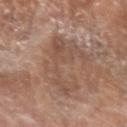| feature | finding |
|---|---|
| notes | catalogued during a skin exam; not biopsied |
| location | the left forearm |
| lesion size | ~7.5 mm (longest diameter) |
| subject | female, aged around 75 |
| illumination | white-light |
| image source | 15 mm crop, total-body photography |